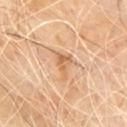The lesion was tiled from a total-body skin photograph and was not biopsied.
Located on the chest.
A 15 mm close-up extracted from a 3D total-body photography capture.
A male subject approximately 70 years of age.